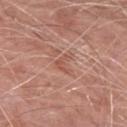The lesion was tiled from a total-body skin photograph and was not biopsied.
A male subject, aged 78 to 82.
Cropped from a total-body skin-imaging series; the visible field is about 15 mm.
The lesion is on the left forearm.